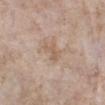Notes:
• workup · no biopsy performed (imaged during a skin exam)
• subject · female, aged approximately 55
• location · the right lower leg
• acquisition · total-body-photography crop, ~15 mm field of view
• TBP lesion metrics · a shape eccentricity near 0.85 and two-axis asymmetry of about 0.5; a mean CIELAB color near L≈59 a*≈15 b*≈29; a classifier nevus-likeness of about 0/100 and a detector confidence of about 100 out of 100 that the crop contains a lesion
• lesion diameter · ≈3 mm